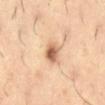{"biopsy_status": "not biopsied; imaged during a skin examination", "lesion_size": {"long_diameter_mm_approx": 3.0}, "site": "abdomen", "image": {"source": "total-body photography crop", "field_of_view_mm": 15}, "patient": {"sex": "male", "age_approx": 55}, "lighting": "cross-polarized"}